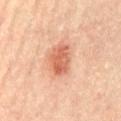Clinical impression: The lesion was photographed on a routine skin check and not biopsied; there is no pathology result. Background: The lesion's longest dimension is about 4 mm. Captured under cross-polarized illumination. Located on the abdomen. The total-body-photography lesion software estimated a lesion color around L≈61 a*≈28 b*≈35 in CIELAB, about 13 CIELAB-L* units darker than the surrounding skin, and a lesion-to-skin contrast of about 8 (normalized; higher = more distinct). The software also gave a detector confidence of about 100 out of 100 that the crop contains a lesion. A male patient aged around 65. Cropped from a whole-body photographic skin survey; the tile spans about 15 mm.